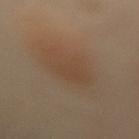Q: Is there a histopathology result?
A: no biopsy performed (imaged during a skin exam)